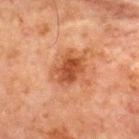The lesion was tiled from a total-body skin photograph and was not biopsied. Longest diameter approximately 4.5 mm. A region of skin cropped from a whole-body photographic capture, roughly 15 mm wide. Imaged with cross-polarized lighting. On the chest. A male subject about 65 years old.